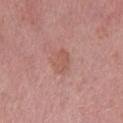Notes:
• follow-up · no biopsy performed (imaged during a skin exam)
• illumination · white-light illumination
• site · the leg
• automated lesion analysis · a lesion area of about 5.5 mm², an outline eccentricity of about 0.7 (0 = round, 1 = elongated), and two-axis asymmetry of about 0.15; border irregularity of about 2 on a 0–10 scale, internal color variation of about 1.5 on a 0–10 scale, and radial color variation of about 0.5; an automated nevus-likeness rating near 0 out of 100 and a detector confidence of about 100 out of 100 that the crop contains a lesion
• patient · female, in their 70s
• imaging modality · 15 mm crop, total-body photography
• lesion size · about 3 mm